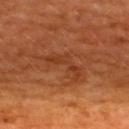Part of a total-body skin-imaging series; this lesion was reviewed on a skin check and was not flagged for biopsy. Approximately 5 mm at its widest. Imaged with cross-polarized lighting. A 15 mm close-up extracted from a 3D total-body photography capture. From the upper back. A female subject in their mid- to late 40s.